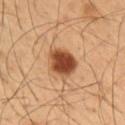No biopsy was performed on this lesion — it was imaged during a full skin examination and was not determined to be concerning. Cropped from a whole-body photographic skin survey; the tile spans about 15 mm. A male subject approximately 55 years of age. Imaged with cross-polarized lighting. Longest diameter approximately 4 mm. On the arm.